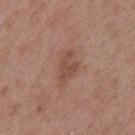Clinical impression: The lesion was photographed on a routine skin check and not biopsied; there is no pathology result. Acquisition and patient details: A male patient, aged approximately 55. Imaged with white-light lighting. A 15 mm close-up tile from a total-body photography series done for melanoma screening. The lesion is located on the mid back. Automated tile analysis of the lesion measured a footprint of about 5.5 mm² and two-axis asymmetry of about 0.4. The software also gave a lesion-to-skin contrast of about 6.5 (normalized; higher = more distinct). And it measured a border-irregularity rating of about 5/10, internal color variation of about 1.5 on a 0–10 scale, and radial color variation of about 0.5. The software also gave a nevus-likeness score of about 0/100 and lesion-presence confidence of about 100/100.